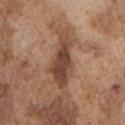No biopsy was performed on this lesion — it was imaged during a full skin examination and was not determined to be concerning.
A region of skin cropped from a whole-body photographic capture, roughly 15 mm wide.
This is a white-light tile.
The patient is a male in their mid- to late 70s.
The lesion is located on the arm.
The lesion's longest dimension is about 6 mm.